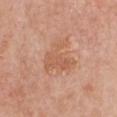Q: Was a biopsy performed?
A: catalogued during a skin exam; not biopsied
Q: What are the patient's age and sex?
A: female, aged 48–52
Q: What is the imaging modality?
A: total-body-photography crop, ~15 mm field of view
Q: Lesion location?
A: the chest